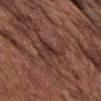Assessment:
The lesion was tiled from a total-body skin photograph and was not biopsied.